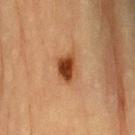Notes:
– workup: no biopsy performed (imaged during a skin exam)
– body site: the left upper arm
– imaging modality: ~15 mm tile from a whole-body skin photo
– patient: male, aged approximately 85 or older
– image-analysis metrics: a mean CIELAB color near L≈39 a*≈23 b*≈34 and about 14 CIELAB-L* units darker than the surrounding skin; a classifier nevus-likeness of about 100/100 and a detector confidence of about 100 out of 100 that the crop contains a lesion
– diameter: about 3.5 mm